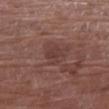Q: Was this lesion biopsied?
A: total-body-photography surveillance lesion; no biopsy
Q: Lesion location?
A: the right upper arm
Q: What are the patient's age and sex?
A: female, aged approximately 70
Q: What kind of image is this?
A: ~15 mm tile from a whole-body skin photo
Q: Illumination type?
A: white-light
Q: What is the lesion's diameter?
A: about 3 mm
Q: What did automated image analysis measure?
A: an average lesion color of about L≈38 a*≈20 b*≈21 (CIELAB), a lesion–skin lightness drop of about 6, and a normalized border contrast of about 5.5; a border-irregularity rating of about 2.5/10, a color-variation rating of about 1.5/10, and a peripheral color-asymmetry measure near 0.5